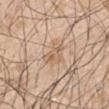| key | value |
|---|---|
| follow-up | catalogued during a skin exam; not biopsied |
| lesion size | ≈3.5 mm |
| subject | male, in their 50s |
| imaging modality | total-body-photography crop, ~15 mm field of view |
| illumination | white-light illumination |
| body site | the right upper arm |